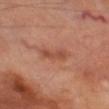Imaged during a routine full-body skin examination; the lesion was not biopsied and no histopathology is available.
A close-up tile cropped from a whole-body skin photograph, about 15 mm across.
The subject is a male in their 70s.
The lesion's longest dimension is about 3.5 mm.
On the left thigh.
Automated tile analysis of the lesion measured a footprint of about 4.5 mm², a shape eccentricity near 0.95, and a symmetry-axis asymmetry near 0.3. It also reported about 8 CIELAB-L* units darker than the surrounding skin and a normalized border contrast of about 6.5. The software also gave border irregularity of about 4 on a 0–10 scale and internal color variation of about 1.5 on a 0–10 scale. And it measured a classifier nevus-likeness of about 5/100 and lesion-presence confidence of about 100/100.